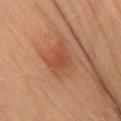{"biopsy_status": "not biopsied; imaged during a skin examination", "lesion_size": {"long_diameter_mm_approx": 3.5}, "lighting": "cross-polarized", "automated_metrics": {"area_mm2_approx": 7.5, "eccentricity": 0.6, "shape_asymmetry": 0.3, "cielab_L": 50, "cielab_a": 27, "cielab_b": 34, "vs_skin_darker_L": 7.0, "vs_skin_contrast_norm": 5.5, "border_irregularity_0_10": 3.5, "color_variation_0_10": 2.0, "peripheral_color_asymmetry": 0.5}, "site": "right thigh", "patient": {"sex": "female", "age_approx": 40}, "image": {"source": "total-body photography crop", "field_of_view_mm": 15}}